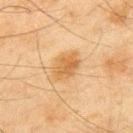Part of a total-body skin-imaging series; this lesion was reviewed on a skin check and was not flagged for biopsy. The lesion is on the upper back. A region of skin cropped from a whole-body photographic capture, roughly 15 mm wide. A male subject approximately 45 years of age. This is a cross-polarized tile. Approximately 4 mm at its widest.